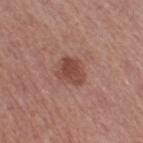Imaged during a routine full-body skin examination; the lesion was not biopsied and no histopathology is available. The recorded lesion diameter is about 3 mm. The lesion is located on the right thigh. A male patient aged 68–72. A region of skin cropped from a whole-body photographic capture, roughly 15 mm wide.